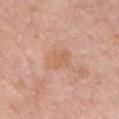workup: imaged on a skin check; not biopsied | subject: female, aged 58–62 | site: the chest | image: total-body-photography crop, ~15 mm field of view.